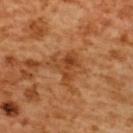biopsy status: total-body-photography surveillance lesion; no biopsy
patient: female, approximately 55 years of age
lesion diameter: ≈4 mm
image: total-body-photography crop, ~15 mm field of view
location: the upper back
automated lesion analysis: a lesion color around L≈46 a*≈27 b*≈40 in CIELAB and about 9 CIELAB-L* units darker than the surrounding skin; border irregularity of about 7 on a 0–10 scale and a within-lesion color-variation index near 5.5/10